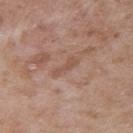The lesion was photographed on a routine skin check and not biopsied; there is no pathology result.
A region of skin cropped from a whole-body photographic capture, roughly 15 mm wide.
This is a white-light tile.
Measured at roughly 3 mm in maximum diameter.
The subject is a male in their 50s.
On the right upper arm.